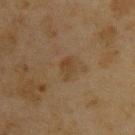{
  "biopsy_status": "not biopsied; imaged during a skin examination",
  "patient": {
    "sex": "male",
    "age_approx": 45
  },
  "lesion_size": {
    "long_diameter_mm_approx": 2.5
  },
  "automated_metrics": {
    "area_mm2_approx": 3.5,
    "eccentricity": 0.7,
    "cielab_L": 33,
    "cielab_a": 13,
    "cielab_b": 27,
    "vs_skin_darker_L": 5.0,
    "vs_skin_contrast_norm": 6.0,
    "border_irregularity_0_10": 3.0,
    "color_variation_0_10": 1.5,
    "peripheral_color_asymmetry": 0.5
  },
  "site": "upper back",
  "image": {
    "source": "total-body photography crop",
    "field_of_view_mm": 15
  },
  "lighting": "cross-polarized"
}